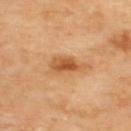Clinical impression:
Part of a total-body skin-imaging series; this lesion was reviewed on a skin check and was not flagged for biopsy.
Context:
A 15 mm crop from a total-body photograph taken for skin-cancer surveillance. About 4 mm across. The tile uses cross-polarized illumination. The lesion is located on the upper back.